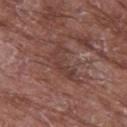workup: no biopsy performed (imaged during a skin exam)
image: 15 mm crop, total-body photography
body site: the left thigh
patient: male, about 65 years old
lighting: white-light illumination
diameter: about 5.5 mm